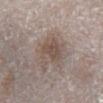This lesion was catalogued during total-body skin photography and was not selected for biopsy.
A 15 mm close-up extracted from a 3D total-body photography capture.
A female patient approximately 60 years of age.
On the left lower leg.
The recorded lesion diameter is about 4 mm.